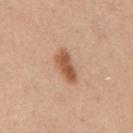Imaged during a routine full-body skin examination; the lesion was not biopsied and no histopathology is available.
A male patient aged 48 to 52.
The total-body-photography lesion software estimated a footprint of about 7 mm², an outline eccentricity of about 0.9 (0 = round, 1 = elongated), and two-axis asymmetry of about 0.25. And it measured a mean CIELAB color near L≈56 a*≈22 b*≈34, about 13 CIELAB-L* units darker than the surrounding skin, and a lesion-to-skin contrast of about 9 (normalized; higher = more distinct). The analysis additionally found a classifier nevus-likeness of about 95/100.
The lesion's longest dimension is about 4.5 mm.
The lesion is on the front of the torso.
A lesion tile, about 15 mm wide, cut from a 3D total-body photograph.